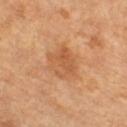biopsy status: catalogued during a skin exam; not biopsied
site: the right thigh
TBP lesion metrics: a footprint of about 11 mm² and an eccentricity of roughly 0.65; a lesion–skin lightness drop of about 8 and a normalized border contrast of about 5.5; border irregularity of about 3 on a 0–10 scale and a within-lesion color-variation index near 4/10
tile lighting: cross-polarized illumination
subject: female, in their mid-50s
image source: ~15 mm tile from a whole-body skin photo